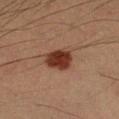Q: Was this lesion biopsied?
A: catalogued during a skin exam; not biopsied
Q: What lighting was used for the tile?
A: cross-polarized
Q: Who is the patient?
A: male, aged 38 to 42
Q: How was this image acquired?
A: ~15 mm crop, total-body skin-cancer survey
Q: What is the anatomic site?
A: the left forearm